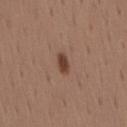Assessment:
The lesion was photographed on a routine skin check and not biopsied; there is no pathology result.
Image and clinical context:
The total-body-photography lesion software estimated a shape eccentricity near 0.85. The software also gave a lesion color around L≈42 a*≈19 b*≈27 in CIELAB, a lesion–skin lightness drop of about 11, and a normalized border contrast of about 9. The analysis additionally found a border-irregularity index near 1.5/10, internal color variation of about 2.5 on a 0–10 scale, and a peripheral color-asymmetry measure near 1. It also reported a nevus-likeness score of about 95/100 and a lesion-detection confidence of about 100/100. A male subject aged 38–42. A lesion tile, about 15 mm wide, cut from a 3D total-body photograph. On the mid back.